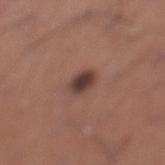Q: Was a biopsy performed?
A: catalogued during a skin exam; not biopsied
Q: Who is the patient?
A: male, aged 43–47
Q: What is the anatomic site?
A: the left lower leg
Q: Automated lesion metrics?
A: an outline eccentricity of about 0.55 (0 = round, 1 = elongated); a border-irregularity rating of about 1.5/10, internal color variation of about 4 on a 0–10 scale, and radial color variation of about 1
Q: Lesion size?
A: about 3 mm
Q: What kind of image is this?
A: 15 mm crop, total-body photography